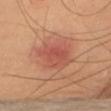Captured during whole-body skin photography for melanoma surveillance; the lesion was not biopsied. From the abdomen. A female subject about 70 years old. The recorded lesion diameter is about 5 mm. A region of skin cropped from a whole-body photographic capture, roughly 15 mm wide. This is a cross-polarized tile.